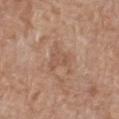follow-up: imaged on a skin check; not biopsied